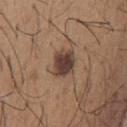Imaged during a routine full-body skin examination; the lesion was not biopsied and no histopathology is available.
The lesion is on the chest.
A male patient aged approximately 65.
Cropped from a whole-body photographic skin survey; the tile spans about 15 mm.